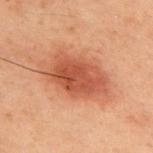Clinical impression:
This lesion was catalogued during total-body skin photography and was not selected for biopsy.
Acquisition and patient details:
The tile uses cross-polarized illumination. A roughly 15 mm field-of-view crop from a total-body skin photograph. The lesion is located on the upper back. The recorded lesion diameter is about 8 mm. The patient is a male about 60 years old.